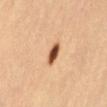The lesion was tiled from a total-body skin photograph and was not biopsied.
Automated image analysis of the tile measured a footprint of about 4.5 mm², a shape eccentricity near 0.8, and a shape-asymmetry score of about 0.25 (0 = symmetric). The analysis additionally found a lesion–skin lightness drop of about 20 and a normalized lesion–skin contrast near 12.5. And it measured a border-irregularity rating of about 2/10, a color-variation rating of about 4/10, and a peripheral color-asymmetry measure near 1.
Cropped from a whole-body photographic skin survey; the tile spans about 15 mm.
The lesion's longest dimension is about 3 mm.
Located on the abdomen.
A female patient aged approximately 65.
Imaged with cross-polarized lighting.